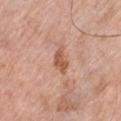{"biopsy_status": "not biopsied; imaged during a skin examination", "patient": {"sex": "male", "age_approx": 80}, "site": "chest", "image": {"source": "total-body photography crop", "field_of_view_mm": 15}}